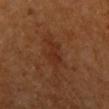A male subject aged 58 to 62. Longest diameter approximately 5 mm. The lesion is located on the left upper arm. The total-body-photography lesion software estimated an area of roughly 8.5 mm², an eccentricity of roughly 0.85, and a symmetry-axis asymmetry near 0.4. It also reported an average lesion color of about L≈29 a*≈21 b*≈29 (CIELAB) and a lesion–skin lightness drop of about 5. A region of skin cropped from a whole-body photographic capture, roughly 15 mm wide.